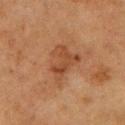<lesion>
<biopsy_status>not biopsied; imaged during a skin examination</biopsy_status>
<site>arm</site>
<patient>
  <sex>female</sex>
  <age_approx>55</age_approx>
</patient>
<lighting>cross-polarized</lighting>
<image>
  <source>total-body photography crop</source>
  <field_of_view_mm>15</field_of_view_mm>
</image>
</lesion>